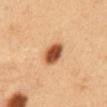Q: Was a biopsy performed?
A: total-body-photography surveillance lesion; no biopsy
Q: What is the imaging modality?
A: ~15 mm tile from a whole-body skin photo
Q: Lesion location?
A: the abdomen
Q: Who is the patient?
A: male, in their 50s
Q: How large is the lesion?
A: ≈3 mm